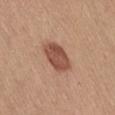anatomic site = the front of the torso | patient = female, aged approximately 55 | diameter = ~4 mm (longest diameter) | lighting = white-light | image source = ~15 mm tile from a whole-body skin photo | automated metrics = a border-irregularity index near 2/10, internal color variation of about 3 on a 0–10 scale, and radial color variation of about 1; a classifier nevus-likeness of about 80/100 and a detector confidence of about 100 out of 100 that the crop contains a lesion.